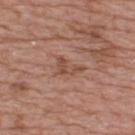Recorded during total-body skin imaging; not selected for excision or biopsy. The recorded lesion diameter is about 3 mm. A roughly 15 mm field-of-view crop from a total-body skin photograph. A male patient aged around 75. The lesion is on the upper back. Imaged with white-light lighting.